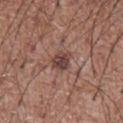{
  "patient": {
    "sex": "male",
    "age_approx": 75
  },
  "lesion_size": {
    "long_diameter_mm_approx": 2.5
  },
  "site": "chest",
  "image": {
    "source": "total-body photography crop",
    "field_of_view_mm": 15
  }
}